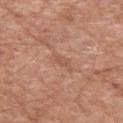Clinical impression: Imaged during a routine full-body skin examination; the lesion was not biopsied and no histopathology is available. Image and clinical context: Located on the arm. A region of skin cropped from a whole-body photographic capture, roughly 15 mm wide. The tile uses white-light illumination. The recorded lesion diameter is about 3.5 mm. Automated tile analysis of the lesion measured an eccentricity of roughly 0.9. The software also gave a mean CIELAB color near L≈55 a*≈22 b*≈31, roughly 6 lightness units darker than nearby skin, and a lesion-to-skin contrast of about 4.5 (normalized; higher = more distinct). It also reported a classifier nevus-likeness of about 0/100 and a lesion-detection confidence of about 100/100. The patient is a male aged approximately 55.